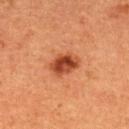Assessment: Part of a total-body skin-imaging series; this lesion was reviewed on a skin check and was not flagged for biopsy. Acquisition and patient details: About 3.5 mm across. A 15 mm close-up extracted from a 3D total-body photography capture. The subject is a female aged 38–42. From the back.